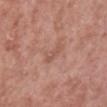follow-up: no biopsy performed (imaged during a skin exam)
TBP lesion metrics: an outline eccentricity of about 0.9 (0 = round, 1 = elongated) and a shape-asymmetry score of about 0.35 (0 = symmetric); about 6 CIELAB-L* units darker than the surrounding skin and a normalized border contrast of about 5; a within-lesion color-variation index near 0/10
illumination: white-light
site: the leg
patient: male, aged 53–57
image: ~15 mm tile from a whole-body skin photo
lesion size: ~2.5 mm (longest diameter)